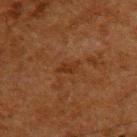{
  "biopsy_status": "not biopsied; imaged during a skin examination",
  "lesion_size": {
    "long_diameter_mm_approx": 3.0
  },
  "image": {
    "source": "total-body photography crop",
    "field_of_view_mm": 15
  },
  "patient": {
    "sex": "male",
    "age_approx": 60
  },
  "site": "upper back",
  "automated_metrics": {
    "area_mm2_approx": 3.0,
    "cielab_L": 25,
    "cielab_a": 18,
    "cielab_b": 27,
    "vs_skin_contrast_norm": 6.0,
    "border_irregularity_0_10": 3.5,
    "peripheral_color_asymmetry": 0.5
  }
}